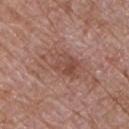Recorded during total-body skin imaging; not selected for excision or biopsy.
On the chest.
The tile uses white-light illumination.
The patient is a male in their 70s.
Cropped from a total-body skin-imaging series; the visible field is about 15 mm.